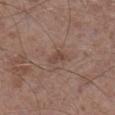Captured during whole-body skin photography for melanoma surveillance; the lesion was not biopsied.
Automated image analysis of the tile measured a lesion color around L≈45 a*≈17 b*≈24 in CIELAB, about 7 CIELAB-L* units darker than the surrounding skin, and a normalized border contrast of about 6. And it measured a detector confidence of about 100 out of 100 that the crop contains a lesion.
On the left lower leg.
A male subject in their mid-40s.
Imaged with white-light lighting.
A region of skin cropped from a whole-body photographic capture, roughly 15 mm wide.
Approximately 2.5 mm at its widest.